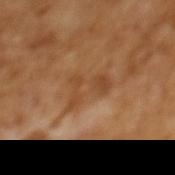Impression:
Recorded during total-body skin imaging; not selected for excision or biopsy.
Acquisition and patient details:
This is a cross-polarized tile. A male subject, aged around 65. Approximately 5.5 mm at its widest. Cropped from a total-body skin-imaging series; the visible field is about 15 mm. Automated tile analysis of the lesion measured an area of roughly 10 mm², an eccentricity of roughly 0.8, and a symmetry-axis asymmetry near 0.5. It also reported an average lesion color of about L≈45 a*≈20 b*≈35 (CIELAB) and about 6 CIELAB-L* units darker than the surrounding skin. It also reported a nevus-likeness score of about 0/100 and a detector confidence of about 95 out of 100 that the crop contains a lesion.